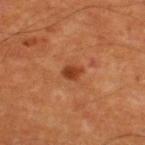| key | value |
|---|---|
| follow-up | no biopsy performed (imaged during a skin exam) |
| tile lighting | cross-polarized |
| image | total-body-photography crop, ~15 mm field of view |
| size | ≈2.5 mm |
| TBP lesion metrics | a lesion area of about 3.5 mm² and two-axis asymmetry of about 0.25; a mean CIELAB color near L≈41 a*≈28 b*≈37, a lesion–skin lightness drop of about 10, and a lesion-to-skin contrast of about 8 (normalized; higher = more distinct) |
| patient | male, aged 53–57 |
| location | the upper back |